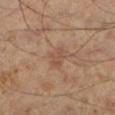Impression: No biopsy was performed on this lesion — it was imaged during a full skin examination and was not determined to be concerning. Acquisition and patient details: Located on the left lower leg. A lesion tile, about 15 mm wide, cut from a 3D total-body photograph. Imaged with cross-polarized lighting. The lesion-visualizer software estimated an average lesion color of about L≈48 a*≈19 b*≈28 (CIELAB), about 6 CIELAB-L* units darker than the surrounding skin, and a lesion-to-skin contrast of about 5 (normalized; higher = more distinct). The software also gave an automated nevus-likeness rating near 0 out of 100 and a detector confidence of about 100 out of 100 that the crop contains a lesion. Measured at roughly 3 mm in maximum diameter. A male patient, aged 53–57.